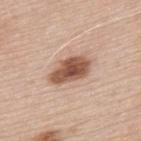Recorded during total-body skin imaging; not selected for excision or biopsy. Located on the upper back. A male patient in their mid- to late 60s. About 5 mm across. A close-up tile cropped from a whole-body skin photograph, about 15 mm across. Automated image analysis of the tile measured an area of roughly 11 mm², a shape eccentricity near 0.85, and two-axis asymmetry of about 0.2.